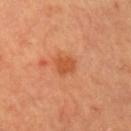{
  "biopsy_status": "not biopsied; imaged during a skin examination",
  "site": "chest",
  "patient": {
    "sex": "male",
    "age_approx": 70
  },
  "image": {
    "source": "total-body photography crop",
    "field_of_view_mm": 15
  }
}